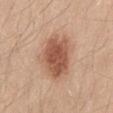notes — total-body-photography surveillance lesion; no biopsy
lighting — white-light
acquisition — ~15 mm tile from a whole-body skin photo
subject — male, in their mid- to late 30s
anatomic site — the mid back
automated metrics — a lesion area of about 17 mm², an outline eccentricity of about 0.7 (0 = round, 1 = elongated), and a symmetry-axis asymmetry near 0.2; border irregularity of about 2.5 on a 0–10 scale, a color-variation rating of about 4/10, and radial color variation of about 1.5
size — ≈5.5 mm